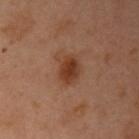Case summary:
• notes · total-body-photography surveillance lesion; no biopsy
• patient · female, aged 53–57
• location · the left arm
• image source · ~15 mm tile from a whole-body skin photo